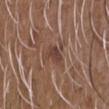biopsy status — no biopsy performed (imaged during a skin exam) | location — the chest | imaging modality — 15 mm crop, total-body photography | patient — male, aged around 50 | size — ≈2.5 mm.